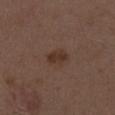The lesion's longest dimension is about 3 mm. Automated image analysis of the tile measured a lesion color around L≈33 a*≈17 b*≈24 in CIELAB, about 7 CIELAB-L* units darker than the surrounding skin, and a normalized border contrast of about 7.5. The lesion is located on the right thigh. A female patient aged 38 to 42. A 15 mm close-up tile from a total-body photography series done for melanoma screening. This is a white-light tile.